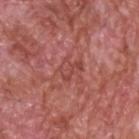The lesion was photographed on a routine skin check and not biopsied; there is no pathology result. A male patient roughly 60 years of age. About 3 mm across. On the head or neck. Captured under white-light illumination. A 15 mm crop from a total-body photograph taken for skin-cancer surveillance.